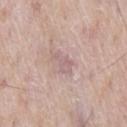notes: total-body-photography surveillance lesion; no biopsy
anatomic site: the chest
patient: male, in their mid-60s
acquisition: ~15 mm tile from a whole-body skin photo